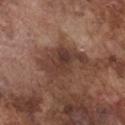Assessment: The lesion was tiled from a total-body skin photograph and was not biopsied. Image and clinical context: A roughly 15 mm field-of-view crop from a total-body skin photograph. The lesion is located on the chest. This is a white-light tile. The subject is a male about 75 years old.